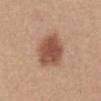Recorded during total-body skin imaging; not selected for excision or biopsy.
This is a white-light tile.
Located on the abdomen.
This image is a 15 mm lesion crop taken from a total-body photograph.
The total-body-photography lesion software estimated a mean CIELAB color near L≈52 a*≈22 b*≈30, a lesion–skin lightness drop of about 13, and a normalized border contrast of about 9. The software also gave border irregularity of about 2 on a 0–10 scale, a within-lesion color-variation index near 3.5/10, and a peripheral color-asymmetry measure near 1.
The subject is a female in their mid- to late 50s.